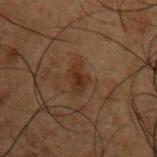{"biopsy_status": "not biopsied; imaged during a skin examination", "automated_metrics": {"cielab_L": 23, "cielab_a": 15, "cielab_b": 22, "vs_skin_darker_L": 6.0, "nevus_likeness_0_100": 35, "lesion_detection_confidence_0_100": 100}, "image": {"source": "total-body photography crop", "field_of_view_mm": 15}, "site": "upper back", "patient": {"sex": "male", "age_approx": 50}, "lesion_size": {"long_diameter_mm_approx": 3.5}, "lighting": "cross-polarized"}